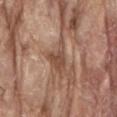Clinical summary:
A lesion tile, about 15 mm wide, cut from a 3D total-body photograph. Located on the back. A male patient aged around 80. Imaged with white-light lighting.